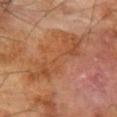Q: Was a biopsy performed?
A: imaged on a skin check; not biopsied
Q: How was the tile lit?
A: cross-polarized
Q: What is the anatomic site?
A: the left forearm
Q: Who is the patient?
A: male, roughly 70 years of age
Q: What is the lesion's diameter?
A: ~9 mm (longest diameter)
Q: What is the imaging modality?
A: total-body-photography crop, ~15 mm field of view